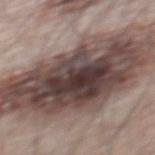{
  "biopsy_status": "not biopsied; imaged during a skin examination",
  "image": {
    "source": "total-body photography crop",
    "field_of_view_mm": 15
  },
  "site": "mid back",
  "patient": {
    "sex": "male",
    "age_approx": 65
  }
}